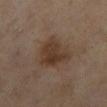Recorded during total-body skin imaging; not selected for excision or biopsy. About 5 mm across. Located on the right lower leg. A 15 mm crop from a total-body photograph taken for skin-cancer surveillance. Automated tile analysis of the lesion measured a lesion area of about 14 mm², an eccentricity of roughly 0.7, and a symmetry-axis asymmetry near 0.35. The analysis additionally found a mean CIELAB color near L≈34 a*≈15 b*≈24. And it measured border irregularity of about 3 on a 0–10 scale and internal color variation of about 3.5 on a 0–10 scale. The patient is a female aged approximately 50. Captured under cross-polarized illumination.